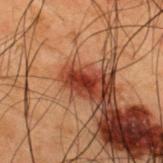notes: no biopsy performed (imaged during a skin exam) | tile lighting: cross-polarized illumination | size: ≈3 mm | patient: male, aged approximately 50 | image-analysis metrics: an area of roughly 4 mm², a shape eccentricity near 0.85, and a shape-asymmetry score of about 0.25 (0 = symmetric); roughly 12 lightness units darker than nearby skin and a lesion-to-skin contrast of about 12 (normalized; higher = more distinct); a border-irregularity index near 2.5/10; an automated nevus-likeness rating near 30 out of 100 and a detector confidence of about 100 out of 100 that the crop contains a lesion | acquisition: 15 mm crop, total-body photography | location: the upper back.